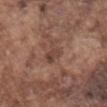notes: no biopsy performed (imaged during a skin exam); site: the abdomen; subject: male, roughly 75 years of age; lesion size: about 3.5 mm; acquisition: ~15 mm crop, total-body skin-cancer survey.